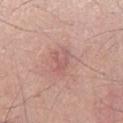Impression:
Part of a total-body skin-imaging series; this lesion was reviewed on a skin check and was not flagged for biopsy.
Acquisition and patient details:
Cropped from a whole-body photographic skin survey; the tile spans about 15 mm. Imaged with white-light lighting. Located on the left lower leg. Longest diameter approximately 3 mm. A male patient roughly 70 years of age.